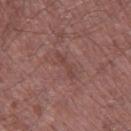Findings:
• follow-up · no biopsy performed (imaged during a skin exam)
• image source · 15 mm crop, total-body photography
• automated lesion analysis · a classifier nevus-likeness of about 0/100
• anatomic site · the leg
• subject · male, aged around 50